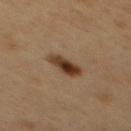Findings:
• follow-up · total-body-photography surveillance lesion; no biopsy
• patient · male, in their 50s
• illumination · cross-polarized
• body site · the mid back
• image source · ~15 mm crop, total-body skin-cancer survey
• image-analysis metrics · a within-lesion color-variation index near 7.5/10 and radial color variation of about 2; a nevus-likeness score of about 100/100